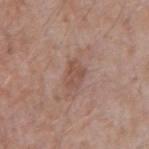{"biopsy_status": "not biopsied; imaged during a skin examination", "patient": {"sex": "male", "age_approx": 55}, "site": "right upper arm", "image": {"source": "total-body photography crop", "field_of_view_mm": 15}, "lighting": "white-light", "lesion_size": {"long_diameter_mm_approx": 3.0}}